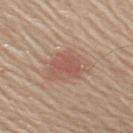Part of a total-body skin-imaging series; this lesion was reviewed on a skin check and was not flagged for biopsy. A lesion tile, about 15 mm wide, cut from a 3D total-body photograph. An algorithmic analysis of the crop reported a lesion color around L≈54 a*≈22 b*≈26 in CIELAB and about 8 CIELAB-L* units darker than the surrounding skin. About 4 mm across. On the right upper arm. Captured under white-light illumination. A male subject, in their 70s.